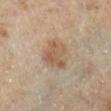Case summary:
– notes — catalogued during a skin exam; not biopsied
– body site — the left lower leg
– imaging modality — 15 mm crop, total-body photography
– subject — female, roughly 60 years of age